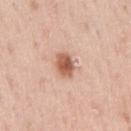Q: Is there a histopathology result?
A: total-body-photography surveillance lesion; no biopsy
Q: Who is the patient?
A: male, aged approximately 40
Q: Illumination type?
A: white-light illumination
Q: What kind of image is this?
A: ~15 mm tile from a whole-body skin photo
Q: What is the anatomic site?
A: the right upper arm
Q: What is the lesion's diameter?
A: ~3 mm (longest diameter)